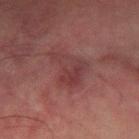Impression: The lesion was photographed on a routine skin check and not biopsied; there is no pathology result. Image and clinical context: From the left forearm. The patient is a male approximately 75 years of age. Cropped from a whole-body photographic skin survey; the tile spans about 15 mm.